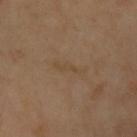Impression:
Part of a total-body skin-imaging series; this lesion was reviewed on a skin check and was not flagged for biopsy.
Image and clinical context:
The lesion is located on the left upper arm. A roughly 15 mm field-of-view crop from a total-body skin photograph. The total-body-photography lesion software estimated a lesion area of about 2.5 mm² and an outline eccentricity of about 0.95 (0 = round, 1 = elongated). And it measured a nevus-likeness score of about 0/100 and lesion-presence confidence of about 100/100. Imaged with cross-polarized lighting. A female patient, aged around 55. The recorded lesion diameter is about 3.5 mm.